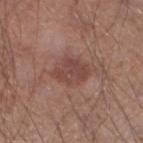Q: Was this lesion biopsied?
A: imaged on a skin check; not biopsied
Q: What kind of image is this?
A: ~15 mm tile from a whole-body skin photo
Q: Where on the body is the lesion?
A: the left lower leg
Q: Patient demographics?
A: male, roughly 55 years of age